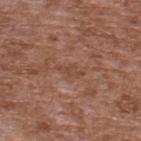Assessment:
Captured during whole-body skin photography for melanoma surveillance; the lesion was not biopsied.
Background:
The subject is a male roughly 75 years of age. Cropped from a total-body skin-imaging series; the visible field is about 15 mm. The lesion is on the upper back.